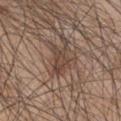Q: Is there a histopathology result?
A: catalogued during a skin exam; not biopsied
Q: Where on the body is the lesion?
A: the right upper arm
Q: Automated lesion metrics?
A: a mean CIELAB color near L≈43 a*≈16 b*≈24, about 9 CIELAB-L* units darker than the surrounding skin, and a normalized lesion–skin contrast near 7.5; internal color variation of about 2.5 on a 0–10 scale and a peripheral color-asymmetry measure near 0.5; an automated nevus-likeness rating near 15 out of 100 and a lesion-detection confidence of about 75/100
Q: What is the imaging modality?
A: ~15 mm tile from a whole-body skin photo
Q: How was the tile lit?
A: white-light
Q: What are the patient's age and sex?
A: male, aged around 45
Q: What is the lesion's diameter?
A: ~4 mm (longest diameter)